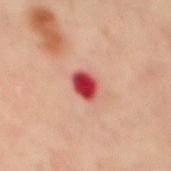| key | value |
|---|---|
| biopsy status | total-body-photography surveillance lesion; no biopsy |
| size | about 2.5 mm |
| body site | the mid back |
| subject | male, approximately 50 years of age |
| tile lighting | cross-polarized illumination |
| image | 15 mm crop, total-body photography |